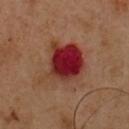Assessment: Recorded during total-body skin imaging; not selected for excision or biopsy. Clinical summary: The subject is a male roughly 50 years of age. Captured under cross-polarized illumination. Located on the chest. Measured at roughly 5.5 mm in maximum diameter. A region of skin cropped from a whole-body photographic capture, roughly 15 mm wide.